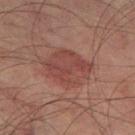Imaged during a routine full-body skin examination; the lesion was not biopsied and no histopathology is available.
The lesion is on the left thigh.
The lesion's longest dimension is about 5.5 mm.
Captured under cross-polarized illumination.
Automated tile analysis of the lesion measured an area of roughly 17 mm², an outline eccentricity of about 0.65 (0 = round, 1 = elongated), and two-axis asymmetry of about 0.2. And it measured a mean CIELAB color near L≈36 a*≈21 b*≈21 and about 6 CIELAB-L* units darker than the surrounding skin.
A male patient, aged around 75.
A 15 mm close-up extracted from a 3D total-body photography capture.